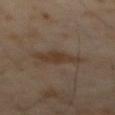This lesion was catalogued during total-body skin photography and was not selected for biopsy. Captured under cross-polarized illumination. A lesion tile, about 15 mm wide, cut from a 3D total-body photograph. The patient is a male aged approximately 65. Measured at roughly 4 mm in maximum diameter.